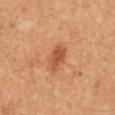biopsy_status: not biopsied; imaged during a skin examination
image:
  source: total-body photography crop
  field_of_view_mm: 15
automated_metrics:
  area_mm2_approx: 5.0
  eccentricity: 0.85
  shape_asymmetry: 0.3
lesion_size:
  long_diameter_mm_approx: 3.0
lighting: cross-polarized
patient:
  sex: male
  age_approx: 60
site: mid back